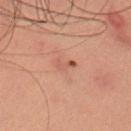<tbp_lesion>
<biopsy_status>not biopsied; imaged during a skin examination</biopsy_status>
<image>
  <source>total-body photography crop</source>
  <field_of_view_mm>15</field_of_view_mm>
</image>
<lighting>cross-polarized</lighting>
<lesion_size>
  <long_diameter_mm_approx>2.5</long_diameter_mm_approx>
</lesion_size>
<site>head or neck</site>
<patient>
  <sex>female</sex>
  <age_approx>20</age_approx>
</patient>
</tbp_lesion>